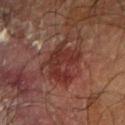The lesion was photographed on a routine skin check and not biopsied; there is no pathology result.
The tile uses cross-polarized illumination.
The lesion is located on the left forearm.
A 15 mm close-up tile from a total-body photography series done for melanoma screening.
Approximately 5 mm at its widest.
A male patient aged around 70.